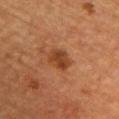* subject — female, aged 68 to 72
* image-analysis metrics — an eccentricity of roughly 0.7 and a symmetry-axis asymmetry near 0.2; a mean CIELAB color near L≈35 a*≈22 b*≈32, about 9 CIELAB-L* units darker than the surrounding skin, and a normalized lesion–skin contrast near 8; border irregularity of about 2 on a 0–10 scale, a color-variation rating of about 2/10, and a peripheral color-asymmetry measure near 0.5
* lesion size — ≈3 mm
* location — the chest
* tile lighting — cross-polarized
* image — ~15 mm crop, total-body skin-cancer survey